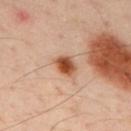Q: What is the anatomic site?
A: the mid back
Q: Patient demographics?
A: male, aged 43 to 47
Q: How was the tile lit?
A: cross-polarized illumination
Q: Lesion size?
A: ≈3 mm
Q: Automated lesion metrics?
A: a within-lesion color-variation index near 6/10 and radial color variation of about 1.5; an automated nevus-likeness rating near 100 out of 100 and a detector confidence of about 100 out of 100 that the crop contains a lesion
Q: How was this image acquired?
A: total-body-photography crop, ~15 mm field of view